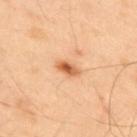Clinical impression: The lesion was tiled from a total-body skin photograph and was not biopsied. Image and clinical context: From the upper back. A male subject approximately 40 years of age. Cropped from a total-body skin-imaging series; the visible field is about 15 mm. Automated tile analysis of the lesion measured an area of roughly 4 mm² and a shape eccentricity near 0.8. It also reported a mean CIELAB color near L≈63 a*≈26 b*≈41 and about 14 CIELAB-L* units darker than the surrounding skin. It also reported a border-irregularity index near 2.5/10. The recorded lesion diameter is about 2.5 mm. The tile uses cross-polarized illumination.